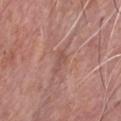biopsy status: no biopsy performed (imaged during a skin exam) | patient: male, aged 58 to 62 | imaging modality: ~15 mm crop, total-body skin-cancer survey | location: the chest.